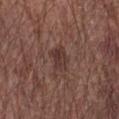Q: Is there a histopathology result?
A: imaged on a skin check; not biopsied
Q: How was the tile lit?
A: white-light illumination
Q: What is the imaging modality?
A: 15 mm crop, total-body photography
Q: What is the lesion's diameter?
A: ~3.5 mm (longest diameter)
Q: What is the anatomic site?
A: the left forearm
Q: Who is the patient?
A: male, in their mid- to late 60s
Q: What did automated image analysis measure?
A: an area of roughly 6 mm², an eccentricity of roughly 0.8, and a symmetry-axis asymmetry near 0.35; a lesion color around L≈36 a*≈19 b*≈20 in CIELAB, about 7 CIELAB-L* units darker than the surrounding skin, and a lesion-to-skin contrast of about 7 (normalized; higher = more distinct); a border-irregularity rating of about 3.5/10 and radial color variation of about 1.5; a nevus-likeness score of about 20/100 and a lesion-detection confidence of about 100/100